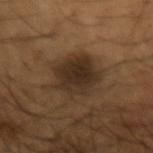Q: Was this lesion biopsied?
A: total-body-photography surveillance lesion; no biopsy
Q: How large is the lesion?
A: ≈5.5 mm
Q: Who is the patient?
A: male, aged around 65
Q: How was this image acquired?
A: total-body-photography crop, ~15 mm field of view
Q: Lesion location?
A: the left forearm